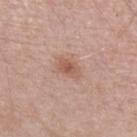- notes: no biopsy performed (imaged during a skin exam)
- imaging modality: 15 mm crop, total-body photography
- body site: the left forearm
- subject: female, aged approximately 45